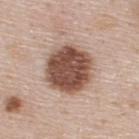Q: Was a biopsy performed?
A: imaged on a skin check; not biopsied
Q: What is the anatomic site?
A: the upper back
Q: What is the imaging modality?
A: 15 mm crop, total-body photography
Q: What is the lesion's diameter?
A: about 5.5 mm
Q: Who is the patient?
A: male, aged around 45
Q: Illumination type?
A: white-light illumination
Q: What did automated image analysis measure?
A: a border-irregularity index near 1.5/10, a color-variation rating of about 4.5/10, and peripheral color asymmetry of about 1.5